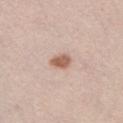  biopsy_status: not biopsied; imaged during a skin examination
  lighting: white-light
  image:
    source: total-body photography crop
    field_of_view_mm: 15
  lesion_size:
    long_diameter_mm_approx: 2.5
  patient:
    sex: male
    age_approx: 30